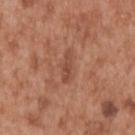Part of a total-body skin-imaging series; this lesion was reviewed on a skin check and was not flagged for biopsy. A male patient, aged approximately 65. The lesion is located on the upper back. The tile uses white-light illumination. Measured at roughly 3 mm in maximum diameter. Cropped from a whole-body photographic skin survey; the tile spans about 15 mm.